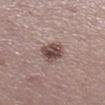Impression:
No biopsy was performed on this lesion — it was imaged during a full skin examination and was not determined to be concerning.
Context:
A female subject aged 48–52. A 15 mm close-up extracted from a 3D total-body photography capture. Longest diameter approximately 3 mm. This is a white-light tile. The lesion is on the right lower leg. The lesion-visualizer software estimated a lesion area of about 7.5 mm², an outline eccentricity of about 0.55 (0 = round, 1 = elongated), and two-axis asymmetry of about 0.2. The analysis additionally found an average lesion color of about L≈47 a*≈17 b*≈19 (CIELAB), about 13 CIELAB-L* units darker than the surrounding skin, and a normalized border contrast of about 9.5. The analysis additionally found a nevus-likeness score of about 75/100 and lesion-presence confidence of about 100/100.